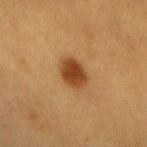Notes:
- workup: imaged on a skin check; not biopsied